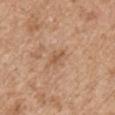follow-up: catalogued during a skin exam; not biopsied | image source: ~15 mm crop, total-body skin-cancer survey | patient: male, aged 53–57 | lighting: white-light | image-analysis metrics: a lesion color around L≈56 a*≈20 b*≈34 in CIELAB and about 8 CIELAB-L* units darker than the surrounding skin; border irregularity of about 3.5 on a 0–10 scale, a color-variation rating of about 1/10, and radial color variation of about 0.5; a lesion-detection confidence of about 100/100 | size: about 2.5 mm | body site: the right upper arm.